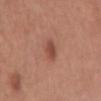biopsy status — no biopsy performed (imaged during a skin exam)
tile lighting — white-light
subject — male, aged approximately 65
site — the mid back
imaging modality — total-body-photography crop, ~15 mm field of view
lesion size — about 3 mm